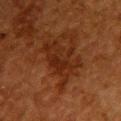{
  "biopsy_status": "not biopsied; imaged during a skin examination",
  "site": "back",
  "patient": {
    "sex": "female",
    "age_approx": 50
  },
  "lesion_size": {
    "long_diameter_mm_approx": 6.5
  },
  "lighting": "cross-polarized",
  "image": {
    "source": "total-body photography crop",
    "field_of_view_mm": 15
  },
  "automated_metrics": {
    "area_mm2_approx": 15.0,
    "eccentricity": 0.8,
    "shape_asymmetry": 0.45,
    "cielab_L": 22,
    "cielab_a": 21,
    "cielab_b": 27,
    "vs_skin_darker_L": 6.0,
    "vs_skin_contrast_norm": 7.5
  }
}